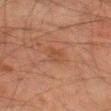Findings:
– biopsy status: catalogued during a skin exam; not biopsied
– acquisition: 15 mm crop, total-body photography
– location: the left thigh
– image-analysis metrics: a border-irregularity rating of about 1.5/10, internal color variation of about 1.5 on a 0–10 scale, and radial color variation of about 0.5
– subject: male, about 80 years old
– illumination: cross-polarized